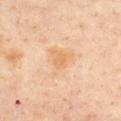Clinical summary: The recorded lesion diameter is about 4 mm. A close-up tile cropped from a whole-body skin photograph, about 15 mm across. A female subject, aged 43–47. The lesion is located on the chest.